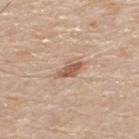Assessment:
Imaged during a routine full-body skin examination; the lesion was not biopsied and no histopathology is available.
Background:
The lesion-visualizer software estimated a footprint of about 5 mm², an eccentricity of roughly 0.85, and a shape-asymmetry score of about 0.25 (0 = symmetric). It also reported a lesion color around L≈58 a*≈19 b*≈31 in CIELAB and a normalized lesion–skin contrast near 7.5. Cropped from a total-body skin-imaging series; the visible field is about 15 mm. The lesion is located on the back. Captured under white-light illumination. A male subject aged 78 to 82. The lesion's longest dimension is about 3.5 mm.